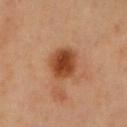Assessment:
No biopsy was performed on this lesion — it was imaged during a full skin examination and was not determined to be concerning.
Image and clinical context:
A lesion tile, about 15 mm wide, cut from a 3D total-body photograph. Approximately 4 mm at its widest. The lesion is located on the chest. Automated tile analysis of the lesion measured an average lesion color of about L≈36 a*≈22 b*≈30 (CIELAB), about 12 CIELAB-L* units darker than the surrounding skin, and a normalized lesion–skin contrast near 10.5. It also reported a nevus-likeness score of about 100/100 and a lesion-detection confidence of about 100/100. The tile uses cross-polarized illumination. A male subject, approximately 55 years of age.